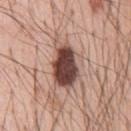No biopsy was performed on this lesion — it was imaged during a full skin examination and was not determined to be concerning. The patient is a male aged 53–57. The tile uses white-light illumination. From the chest. A roughly 15 mm field-of-view crop from a total-body skin photograph. Measured at roughly 6.5 mm in maximum diameter.